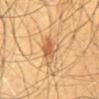No biopsy was performed on this lesion — it was imaged during a full skin examination and was not determined to be concerning. A region of skin cropped from a whole-body photographic capture, roughly 15 mm wide. On the front of the torso. About 3 mm across. Automated image analysis of the tile measured a footprint of about 7 mm², an outline eccentricity of about 0.4 (0 = round, 1 = elongated), and two-axis asymmetry of about 0.25. And it measured a lesion color around L≈55 a*≈21 b*≈37 in CIELAB, about 9 CIELAB-L* units darker than the surrounding skin, and a lesion-to-skin contrast of about 6.5 (normalized; higher = more distinct). The analysis additionally found a nevus-likeness score of about 85/100 and a detector confidence of about 100 out of 100 that the crop contains a lesion. Captured under cross-polarized illumination. A male subject, in their 60s.